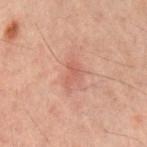Part of a total-body skin-imaging series; this lesion was reviewed on a skin check and was not flagged for biopsy. A male patient about 60 years old. Imaged with cross-polarized lighting. From the front of the torso. The recorded lesion diameter is about 2.5 mm. Automated image analysis of the tile measured an area of roughly 3.5 mm² and an outline eccentricity of about 0.7 (0 = round, 1 = elongated). The software also gave a border-irregularity index near 3/10, a within-lesion color-variation index near 1/10, and peripheral color asymmetry of about 0.5. The analysis additionally found a detector confidence of about 100 out of 100 that the crop contains a lesion. Cropped from a total-body skin-imaging series; the visible field is about 15 mm.